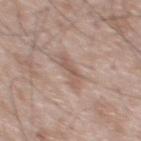Recorded during total-body skin imaging; not selected for excision or biopsy. A 15 mm close-up extracted from a 3D total-body photography capture. The lesion's longest dimension is about 4 mm. Captured under white-light illumination. The subject is a male aged 63 to 67. On the chest.